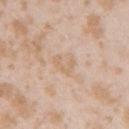biopsy_status: not biopsied; imaged during a skin examination
lighting: white-light
lesion_size:
  long_diameter_mm_approx: 3.0
site: right upper arm
patient:
  sex: female
  age_approx: 25
image:
  source: total-body photography crop
  field_of_view_mm: 15